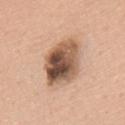Recorded during total-body skin imaging; not selected for excision or biopsy. The subject is a male in their mid-50s. Captured under white-light illumination. A 15 mm crop from a total-body photograph taken for skin-cancer surveillance. The lesion is located on the upper back.